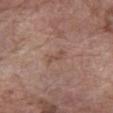This lesion was catalogued during total-body skin photography and was not selected for biopsy.
From the left forearm.
The lesion-visualizer software estimated a mean CIELAB color near L≈49 a*≈19 b*≈25, a lesion–skin lightness drop of about 7, and a normalized border contrast of about 5. And it measured a border-irregularity rating of about 4/10 and internal color variation of about 0 on a 0–10 scale. It also reported an automated nevus-likeness rating near 0 out of 100 and lesion-presence confidence of about 100/100.
A roughly 15 mm field-of-view crop from a total-body skin photograph.
A male patient aged around 60.
Approximately 2.5 mm at its widest.
Imaged with white-light lighting.